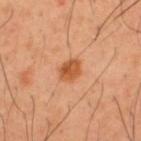workup — imaged on a skin check; not biopsied.